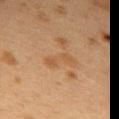Q: Was a biopsy performed?
A: no biopsy performed (imaged during a skin exam)
Q: What kind of image is this?
A: ~15 mm crop, total-body skin-cancer survey
Q: What are the patient's age and sex?
A: female, aged around 40
Q: Illumination type?
A: cross-polarized illumination
Q: Lesion location?
A: the upper back